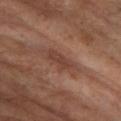Impression: Part of a total-body skin-imaging series; this lesion was reviewed on a skin check and was not flagged for biopsy. Context: Imaged with cross-polarized lighting. About 4 mm across. On the right lower leg. Cropped from a total-body skin-imaging series; the visible field is about 15 mm. A female patient, approximately 65 years of age.